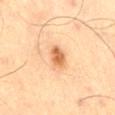<lesion>
  <biopsy_status>not biopsied; imaged during a skin examination</biopsy_status>
  <automated_metrics>
    <area_mm2_approx>4.5</area_mm2_approx>
    <shape_asymmetry>0.15</shape_asymmetry>
    <cielab_L>54</cielab_L>
    <cielab_a>21</cielab_a>
    <cielab_b>36</cielab_b>
    <peripheral_color_asymmetry>1.0</peripheral_color_asymmetry>
    <nevus_likeness_0_100>95</nevus_likeness_0_100>
  </automated_metrics>
  <patient>
    <sex>male</sex>
    <age_approx>70</age_approx>
  </patient>
  <lesion_size>
    <long_diameter_mm_approx>2.5</long_diameter_mm_approx>
  </lesion_size>
  <site>right thigh</site>
  <lighting>cross-polarized</lighting>
  <image>
    <source>total-body photography crop</source>
    <field_of_view_mm>15</field_of_view_mm>
  </image>
</lesion>